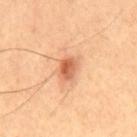Q: Was a biopsy performed?
A: catalogued during a skin exam; not biopsied
Q: What are the patient's age and sex?
A: male, aged 48 to 52
Q: What lighting was used for the tile?
A: cross-polarized illumination
Q: How large is the lesion?
A: ≈3.5 mm
Q: What is the imaging modality?
A: 15 mm crop, total-body photography
Q: Lesion location?
A: the mid back